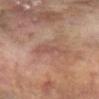Q: Was a biopsy performed?
A: total-body-photography surveillance lesion; no biopsy
Q: Lesion size?
A: ≈3.5 mm
Q: What is the imaging modality?
A: 15 mm crop, total-body photography
Q: Automated lesion metrics?
A: an average lesion color of about L≈46 a*≈20 b*≈23 (CIELAB), about 6 CIELAB-L* units darker than the surrounding skin, and a lesion-to-skin contrast of about 5 (normalized; higher = more distinct)
Q: Where on the body is the lesion?
A: the right arm
Q: Patient demographics?
A: female, aged around 80